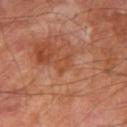illumination: cross-polarized
acquisition: total-body-photography crop, ~15 mm field of view
lesion size: ~2.5 mm (longest diameter)
automated lesion analysis: a lesion area of about 3 mm², an eccentricity of roughly 0.8, and a shape-asymmetry score of about 0.5 (0 = symmetric)
body site: the right thigh
subject: male, aged around 70